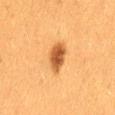Assessment:
Imaged during a routine full-body skin examination; the lesion was not biopsied and no histopathology is available.
Background:
This image is a 15 mm lesion crop taken from a total-body photograph. The lesion is on the back. A female subject aged around 40.